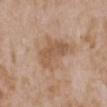Assessment:
No biopsy was performed on this lesion — it was imaged during a full skin examination and was not determined to be concerning.
Image and clinical context:
The total-body-photography lesion software estimated a border-irregularity rating of about 4.5/10 and a peripheral color-asymmetry measure near 1. It also reported a nevus-likeness score of about 10/100 and a lesion-detection confidence of about 100/100. A female patient, in their mid- to late 30s. The recorded lesion diameter is about 5.5 mm. A roughly 15 mm field-of-view crop from a total-body skin photograph. The tile uses white-light illumination. The lesion is located on the left upper arm.